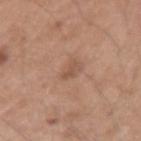Clinical impression:
This lesion was catalogued during total-body skin photography and was not selected for biopsy.
Context:
Captured under white-light illumination. Automated tile analysis of the lesion measured an area of roughly 2 mm², an outline eccentricity of about 0.9 (0 = round, 1 = elongated), and two-axis asymmetry of about 0.45. And it measured a lesion-to-skin contrast of about 5.5 (normalized; higher = more distinct). A male subject aged 48–52. From the left upper arm. A 15 mm close-up tile from a total-body photography series done for melanoma screening. The lesion's longest dimension is about 2.5 mm.